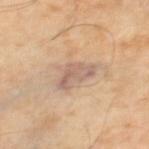Clinical impression: Recorded during total-body skin imaging; not selected for excision or biopsy. Clinical summary: Cropped from a total-body skin-imaging series; the visible field is about 15 mm. A male subject, about 55 years old. Measured at roughly 4.5 mm in maximum diameter. Captured under cross-polarized illumination. From the right thigh.